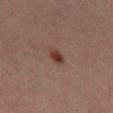A male patient in their 30s. A 15 mm crop from a total-body photograph taken for skin-cancer surveillance. The lesion is located on the abdomen.